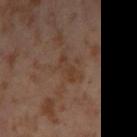automated metrics — an area of roughly 4.5 mm² and an eccentricity of roughly 0.9; a peripheral color-asymmetry measure near 0.5; a classifier nevus-likeness of about 0/100 and a lesion-detection confidence of about 100/100 | acquisition — ~15 mm crop, total-body skin-cancer survey | location — the left thigh | diameter — ≈3.5 mm | illumination — cross-polarized | subject — female, aged around 55.